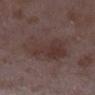biopsy_status: not biopsied; imaged during a skin examination
patient:
  sex: female
  age_approx: 35
site: right lower leg
image:
  source: total-body photography crop
  field_of_view_mm: 15